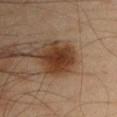Clinical impression:
The lesion was photographed on a routine skin check and not biopsied; there is no pathology result.
Clinical summary:
The lesion is on the chest. Imaged with cross-polarized lighting. The patient is a male approximately 35 years of age. A lesion tile, about 15 mm wide, cut from a 3D total-body photograph. Longest diameter approximately 3.5 mm.